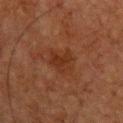image source=15 mm crop, total-body photography | body site=the chest | size=≈3.5 mm | TBP lesion metrics=a footprint of about 7 mm²; a lesion color around L≈25 a*≈19 b*≈26 in CIELAB and a lesion–skin lightness drop of about 5; a border-irregularity index near 4.5/10 and a peripheral color-asymmetry measure near 0.5; a nevus-likeness score of about 0/100 | tile lighting=cross-polarized illumination | patient=male, aged approximately 50.